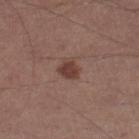biopsy status = no biopsy performed (imaged during a skin exam) | acquisition = ~15 mm crop, total-body skin-cancer survey | patient = male, aged 53–57 | anatomic site = the leg.